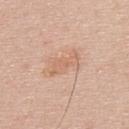• notes · no biopsy performed (imaged during a skin exam)
• site · the upper back
• image · ~15 mm tile from a whole-body skin photo
• subject · male, about 45 years old
• diameter · ≈4.5 mm